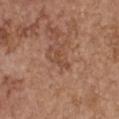notes: imaged on a skin check; not biopsied
anatomic site: the chest
automated metrics: border irregularity of about 7 on a 0–10 scale, a within-lesion color-variation index near 0/10, and radial color variation of about 0; a nevus-likeness score of about 0/100 and lesion-presence confidence of about 100/100
lesion diameter: ~3 mm (longest diameter)
tile lighting: white-light illumination
subject: female, about 65 years old
image: total-body-photography crop, ~15 mm field of view